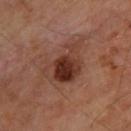Background:
A 15 mm close-up extracted from a 3D total-body photography capture. The lesion is on the upper back. A male patient, roughly 65 years of age. The lesion's longest dimension is about 5.5 mm.